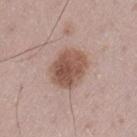Impression: The lesion was photographed on a routine skin check and not biopsied; there is no pathology result. Acquisition and patient details: Approximately 4.5 mm at its widest. The lesion is on the left thigh. A male subject, aged approximately 50. Automated tile analysis of the lesion measured a border-irregularity index near 1.5/10 and radial color variation of about 1.5. The analysis additionally found a classifier nevus-likeness of about 75/100 and a lesion-detection confidence of about 100/100. Captured under white-light illumination. A region of skin cropped from a whole-body photographic capture, roughly 15 mm wide.